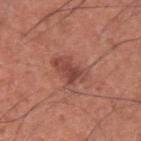| field | value |
|---|---|
| biopsy status | imaged on a skin check; not biopsied |
| patient | male, approximately 35 years of age |
| automated metrics | a lesion area of about 8.5 mm² and a symmetry-axis asymmetry near 0.35 |
| image source | ~15 mm crop, total-body skin-cancer survey |
| body site | the upper back |